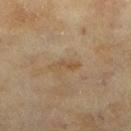Clinical impression:
Imaged during a routine full-body skin examination; the lesion was not biopsied and no histopathology is available.
Clinical summary:
Cropped from a whole-body photographic skin survey; the tile spans about 15 mm. Imaged with cross-polarized lighting. The lesion is located on the left lower leg. The patient is a female aged 58–62. Longest diameter approximately 3.5 mm.